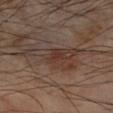follow-up — catalogued during a skin exam; not biopsied
lesion diameter — ≈6.5 mm
patient — male, in their mid- to late 60s
image — ~15 mm crop, total-body skin-cancer survey
lighting — cross-polarized
body site — the left forearm